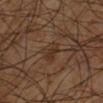biopsy_status: not biopsied; imaged during a skin examination
lighting: cross-polarized
patient:
  sex: male
  age_approx: 60
site: left lower leg
automated_metrics:
  area_mm2_approx: 4.5
  eccentricity: 0.65
  cielab_L: 30
  cielab_a: 16
  cielab_b: 26
  vs_skin_darker_L: 5.0
  vs_skin_contrast_norm: 6.0
  lesion_detection_confidence_0_100: 100
lesion_size:
  long_diameter_mm_approx: 2.5
image:
  source: total-body photography crop
  field_of_view_mm: 15